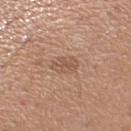biopsy_status: not biopsied; imaged during a skin examination
site: right upper arm
patient:
  sex: male
  age_approx: 40
image:
  source: total-body photography crop
  field_of_view_mm: 15